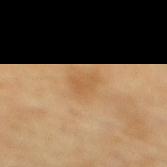No biopsy was performed on this lesion — it was imaged during a full skin examination and was not determined to be concerning. On the mid back. The patient is a male approximately 70 years of age. Captured under cross-polarized illumination. The total-body-photography lesion software estimated a lesion color around L≈54 a*≈18 b*≈38 in CIELAB, about 6 CIELAB-L* units darker than the surrounding skin, and a normalized border contrast of about 4.5. It also reported a nevus-likeness score of about 0/100 and a detector confidence of about 100 out of 100 that the crop contains a lesion. A 15 mm close-up tile from a total-body photography series done for melanoma screening. The lesion's longest dimension is about 3 mm.